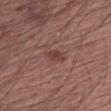The lesion was photographed on a routine skin check and not biopsied; there is no pathology result. A male patient aged 63–67. Approximately 2.5 mm at its widest. The lesion is located on the left upper arm. Captured under white-light illumination. A close-up tile cropped from a whole-body skin photograph, about 15 mm across.